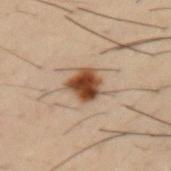biopsy status = imaged on a skin check; not biopsied | imaging modality = 15 mm crop, total-body photography | patient = male, approximately 30 years of age | lighting = cross-polarized | size = ~3.5 mm (longest diameter) | body site = the left upper arm | TBP lesion metrics = an area of roughly 8.5 mm²; a within-lesion color-variation index near 5/10 and peripheral color asymmetry of about 1.5; an automated nevus-likeness rating near 100 out of 100 and a lesion-detection confidence of about 100/100.